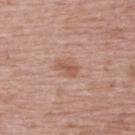No biopsy was performed on this lesion — it was imaged during a full skin examination and was not determined to be concerning. The lesion is located on the upper back. Captured under white-light illumination. A 15 mm close-up tile from a total-body photography series done for melanoma screening. A female subject roughly 65 years of age.